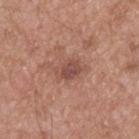Clinical impression:
The lesion was tiled from a total-body skin photograph and was not biopsied.
Clinical summary:
The lesion-visualizer software estimated border irregularity of about 3 on a 0–10 scale, a color-variation rating of about 3.5/10, and a peripheral color-asymmetry measure near 1. It also reported an automated nevus-likeness rating near 10 out of 100 and a detector confidence of about 100 out of 100 that the crop contains a lesion. The tile uses white-light illumination. Longest diameter approximately 3.5 mm. On the back. A 15 mm close-up extracted from a 3D total-body photography capture. A male subject, aged 53 to 57.